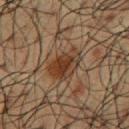No biopsy was performed on this lesion — it was imaged during a full skin examination and was not determined to be concerning. From the chest. Longest diameter approximately 4.5 mm. A close-up tile cropped from a whole-body skin photograph, about 15 mm across. A male patient, roughly 60 years of age. Captured under cross-polarized illumination.